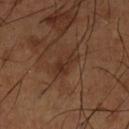Captured during whole-body skin photography for melanoma surveillance; the lesion was not biopsied. From the left lower leg. A roughly 15 mm field-of-view crop from a total-body skin photograph. Measured at roughly 4 mm in maximum diameter. Automated tile analysis of the lesion measured a lesion area of about 6 mm² and an eccentricity of roughly 0.85. Captured under cross-polarized illumination. A male subject, approximately 65 years of age.